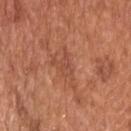No biopsy was performed on this lesion — it was imaged during a full skin examination and was not determined to be concerning. A male patient aged approximately 65. From the upper back. Captured under white-light illumination. A 15 mm crop from a total-body photograph taken for skin-cancer surveillance. The total-body-photography lesion software estimated a border-irregularity index near 3.5/10, a color-variation rating of about 1.5/10, and radial color variation of about 0.5. About 2.5 mm across.